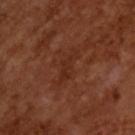  patient:
    sex: male
    age_approx: 65
  image:
    source: total-body photography crop
    field_of_view_mm: 15